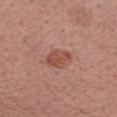Q: How was this image acquired?
A: ~15 mm tile from a whole-body skin photo
Q: Illumination type?
A: white-light
Q: What is the lesion's diameter?
A: about 3 mm
Q: Who is the patient?
A: female, roughly 55 years of age
Q: What is the anatomic site?
A: the right forearm